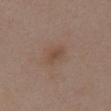The lesion was tiled from a total-body skin photograph and was not biopsied. A close-up tile cropped from a whole-body skin photograph, about 15 mm across. From the chest. Approximately 3 mm at its widest. The subject is a female in their mid- to late 50s. Automated tile analysis of the lesion measured a lesion area of about 4.5 mm², an eccentricity of roughly 0.75, and a shape-asymmetry score of about 0.2 (0 = symmetric). And it measured a mean CIELAB color near L≈47 a*≈17 b*≈27, a lesion–skin lightness drop of about 6, and a normalized lesion–skin contrast near 5.5.